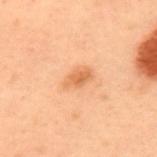<case>
<biopsy_status>not biopsied; imaged during a skin examination</biopsy_status>
<lighting>cross-polarized</lighting>
<image>
  <source>total-body photography crop</source>
  <field_of_view_mm>15</field_of_view_mm>
</image>
<patient>
  <sex>male</sex>
  <age_approx>55</age_approx>
</patient>
<site>upper back</site>
<lesion_size>
  <long_diameter_mm_approx>3.5</long_diameter_mm_approx>
</lesion_size>
<automated_metrics>
  <eccentricity>0.85</eccentricity>
  <cielab_L>54</cielab_L>
  <cielab_a>22</cielab_a>
  <cielab_b>35</cielab_b>
  <vs_skin_contrast_norm>6.5</vs_skin_contrast_norm>
  <border_irregularity_0_10>2.0</border_irregularity_0_10>
  <color_variation_0_10>2.5</color_variation_0_10>
  <nevus_likeness_0_100>80</nevus_likeness_0_100>
  <lesion_detection_confidence_0_100>100</lesion_detection_confidence_0_100>
</automated_metrics>
</case>